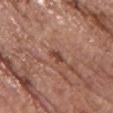This lesion was catalogued during total-body skin photography and was not selected for biopsy. A region of skin cropped from a whole-body photographic capture, roughly 15 mm wide. Automated image analysis of the tile measured a footprint of about 2.5 mm², an outline eccentricity of about 0.9 (0 = round, 1 = elongated), and two-axis asymmetry of about 0.4. It also reported an average lesion color of about L≈44 a*≈23 b*≈27 (CIELAB) and a lesion–skin lightness drop of about 10. It also reported a border-irregularity index near 4/10, a within-lesion color-variation index near 0/10, and radial color variation of about 0. It also reported an automated nevus-likeness rating near 0 out of 100 and a lesion-detection confidence of about 100/100. A female patient, aged 63–67. The lesion is located on the chest. This is a white-light tile.